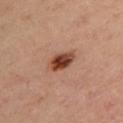Case summary:
• notes — imaged on a skin check; not biopsied
• subject — female, in their 40s
• location — the upper back
• illumination — cross-polarized illumination
• imaging modality — ~15 mm crop, total-body skin-cancer survey
• automated lesion analysis — an average lesion color of about L≈42 a*≈25 b*≈30 (CIELAB), roughly 16 lightness units darker than nearby skin, and a normalized lesion–skin contrast near 12
• lesion size — about 3.5 mm